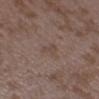| key | value |
|---|---|
| follow-up | no biopsy performed (imaged during a skin exam) |
| body site | the right forearm |
| lesion diameter | ≈2.5 mm |
| image | ~15 mm tile from a whole-body skin photo |
| subject | female, aged around 35 |
| TBP lesion metrics | an area of roughly 4 mm² and an eccentricity of roughly 0.65; a lesion color around L≈45 a*≈14 b*≈23 in CIELAB and a normalized border contrast of about 4.5; a detector confidence of about 100 out of 100 that the crop contains a lesion |
| lighting | white-light |